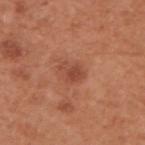No biopsy was performed on this lesion — it was imaged during a full skin examination and was not determined to be concerning. Longest diameter approximately 3 mm. A close-up tile cropped from a whole-body skin photograph, about 15 mm across. From the back. A male patient aged approximately 55. The total-body-photography lesion software estimated a shape-asymmetry score of about 0.25 (0 = symmetric). The analysis additionally found a classifier nevus-likeness of about 25/100. Imaged with white-light lighting.